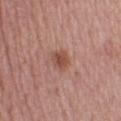Q: Was a biopsy performed?
A: imaged on a skin check; not biopsied
Q: What kind of image is this?
A: total-body-photography crop, ~15 mm field of view
Q: Lesion location?
A: the left lower leg
Q: Lesion size?
A: about 2.5 mm
Q: What are the patient's age and sex?
A: female, roughly 50 years of age
Q: What lighting was used for the tile?
A: white-light